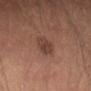Findings:
- follow-up — imaged on a skin check; not biopsied
- image source — ~15 mm tile from a whole-body skin photo
- location — the lower back
- patient — male, in their mid- to late 30s
- size — about 3 mm
- lighting — white-light
- automated lesion analysis — a lesion area of about 5.5 mm², an outline eccentricity of about 0.7 (0 = round, 1 = elongated), and a shape-asymmetry score of about 0.25 (0 = symmetric); a mean CIELAB color near L≈41 a*≈21 b*≈25, about 8 CIELAB-L* units darker than the surrounding skin, and a normalized border contrast of about 7